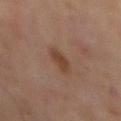Assessment: Recorded during total-body skin imaging; not selected for excision or biopsy. Acquisition and patient details: A 15 mm close-up tile from a total-body photography series done for melanoma screening. This is a cross-polarized tile. The patient is a male approximately 65 years of age. The recorded lesion diameter is about 3.5 mm. The lesion is on the mid back.